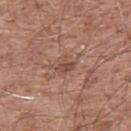imaging modality: 15 mm crop, total-body photography
anatomic site: the upper back
lesion size: ≈2.5 mm
illumination: white-light
TBP lesion metrics: a border-irregularity index near 3.5/10, a color-variation rating of about 3/10, and a peripheral color-asymmetry measure near 1; a lesion-detection confidence of about 100/100
patient: male, aged approximately 55